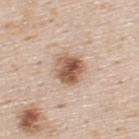The lesion was photographed on a routine skin check and not biopsied; there is no pathology result.
Approximately 3.5 mm at its widest.
The patient is a male in their mid-40s.
On the upper back.
Automated image analysis of the tile measured a lesion color around L≈57 a*≈19 b*≈29 in CIELAB, about 15 CIELAB-L* units darker than the surrounding skin, and a normalized border contrast of about 10. It also reported border irregularity of about 2 on a 0–10 scale, internal color variation of about 8 on a 0–10 scale, and a peripheral color-asymmetry measure near 3. And it measured a classifier nevus-likeness of about 90/100 and a detector confidence of about 100 out of 100 that the crop contains a lesion.
The tile uses white-light illumination.
A roughly 15 mm field-of-view crop from a total-body skin photograph.